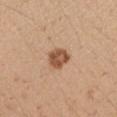Notes:
* workup — total-body-photography surveillance lesion; no biopsy
* anatomic site — the right upper arm
* lighting — white-light illumination
* size — about 3 mm
* image-analysis metrics — a lesion area of about 6.5 mm², an outline eccentricity of about 0.65 (0 = round, 1 = elongated), and a shape-asymmetry score of about 0.2 (0 = symmetric); an average lesion color of about L≈53 a*≈21 b*≈33 (CIELAB), about 14 CIELAB-L* units darker than the surrounding skin, and a normalized lesion–skin contrast near 9.5; a classifier nevus-likeness of about 75/100
* patient — female, aged 28–32
* image source — total-body-photography crop, ~15 mm field of view